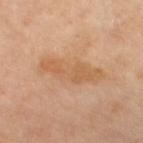Q: Was this lesion biopsied?
A: imaged on a skin check; not biopsied
Q: How large is the lesion?
A: ≈7.5 mm
Q: Who is the patient?
A: female, aged 68–72
Q: Where on the body is the lesion?
A: the left lower leg
Q: How was this image acquired?
A: ~15 mm crop, total-body skin-cancer survey